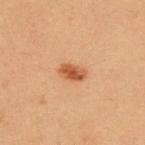notes = imaged on a skin check; not biopsied
image source = ~15 mm tile from a whole-body skin photo
patient = female, aged 28–32
lesion size = about 3 mm
location = the upper back
illumination = cross-polarized